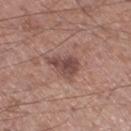Recorded during total-body skin imaging; not selected for excision or biopsy.